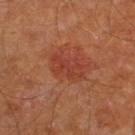follow-up: imaged on a skin check; not biopsied
body site: the right thigh
TBP lesion metrics: an average lesion color of about L≈39 a*≈29 b*≈30 (CIELAB) and a lesion-to-skin contrast of about 5.5 (normalized; higher = more distinct)
size: about 4 mm
imaging modality: ~15 mm crop, total-body skin-cancer survey
patient: male, aged approximately 65